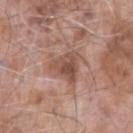Q: Was a biopsy performed?
A: total-body-photography surveillance lesion; no biopsy
Q: What lighting was used for the tile?
A: white-light
Q: What kind of image is this?
A: ~15 mm tile from a whole-body skin photo
Q: Where on the body is the lesion?
A: the left forearm
Q: Who is the patient?
A: male, aged approximately 75
Q: How large is the lesion?
A: ≈4.5 mm